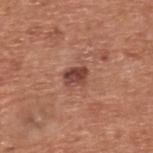Q: Is there a histopathology result?
A: no biopsy performed (imaged during a skin exam)
Q: Automated lesion metrics?
A: a mean CIELAB color near L≈43 a*≈24 b*≈26, a lesion–skin lightness drop of about 13, and a normalized border contrast of about 10; a border-irregularity index near 2/10, internal color variation of about 4.5 on a 0–10 scale, and radial color variation of about 1.5; a nevus-likeness score of about 50/100 and a lesion-detection confidence of about 100/100
Q: What is the imaging modality?
A: total-body-photography crop, ~15 mm field of view
Q: Lesion size?
A: ~2.5 mm (longest diameter)
Q: Who is the patient?
A: male, in their mid-50s
Q: Lesion location?
A: the upper back